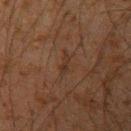This lesion was catalogued during total-body skin photography and was not selected for biopsy. Cropped from a whole-body photographic skin survey; the tile spans about 15 mm. The recorded lesion diameter is about 2.5 mm. Captured under cross-polarized illumination. The patient is a male roughly 35 years of age. The lesion is on the left upper arm.